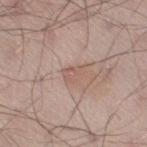The lesion was tiled from a total-body skin photograph and was not biopsied.
The tile uses white-light illumination.
A male subject approximately 30 years of age.
A region of skin cropped from a whole-body photographic capture, roughly 15 mm wide.
From the right thigh.